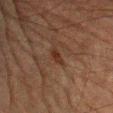{"biopsy_status": "not biopsied; imaged during a skin examination", "automated_metrics": {"cielab_L": 24, "cielab_a": 17, "cielab_b": 22, "vs_skin_darker_L": 6.0, "vs_skin_contrast_norm": 7.5}, "site": "left upper arm", "patient": {"sex": "male", "age_approx": 60}, "image": {"source": "total-body photography crop", "field_of_view_mm": 15}, "lesion_size": {"long_diameter_mm_approx": 2.5}, "lighting": "cross-polarized"}